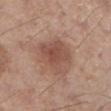Imaged during a routine full-body skin examination; the lesion was not biopsied and no histopathology is available. An algorithmic analysis of the crop reported a lesion area of about 17 mm², an outline eccentricity of about 0.55 (0 = round, 1 = elongated), and two-axis asymmetry of about 0.25. The analysis additionally found internal color variation of about 4 on a 0–10 scale and radial color variation of about 1. The lesion is on the left lower leg. Imaged with white-light lighting. The patient is a male aged 53 to 57. A 15 mm crop from a total-body photograph taken for skin-cancer surveillance. The lesion's longest dimension is about 5 mm.